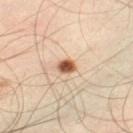Assessment:
The lesion was photographed on a routine skin check and not biopsied; there is no pathology result.
Image and clinical context:
About 2 mm across. A male patient aged approximately 35. On the right leg. The tile uses cross-polarized illumination. A 15 mm crop from a total-body photograph taken for skin-cancer surveillance.